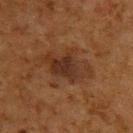Recorded during total-body skin imaging; not selected for excision or biopsy. Automated tile analysis of the lesion measured a footprint of about 14 mm² and an outline eccentricity of about 0.85 (0 = round, 1 = elongated). The analysis additionally found roughly 7 lightness units darker than nearby skin and a lesion-to-skin contrast of about 8 (normalized; higher = more distinct). It also reported an automated nevus-likeness rating near 25 out of 100 and a lesion-detection confidence of about 100/100. From the upper back. A male subject about 60 years old. The recorded lesion diameter is about 6 mm. Captured under cross-polarized illumination. A 15 mm close-up extracted from a 3D total-body photography capture.